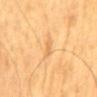Findings:
• notes · catalogued during a skin exam; not biopsied
• acquisition · 15 mm crop, total-body photography
• size · about 2.5 mm
• patient · male, in their 60s
• automated metrics · a mean CIELAB color near L≈70 a*≈21 b*≈47, a lesion–skin lightness drop of about 7, and a lesion-to-skin contrast of about 5 (normalized; higher = more distinct); border irregularity of about 4 on a 0–10 scale and a color-variation rating of about 0/10
• location · the mid back